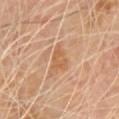| field | value |
|---|---|
| follow-up | no biopsy performed (imaged during a skin exam) |
| lesion size | about 3 mm |
| TBP lesion metrics | a nevus-likeness score of about 0/100 and a lesion-detection confidence of about 100/100 |
| lighting | cross-polarized |
| image source | ~15 mm tile from a whole-body skin photo |
| location | the chest |
| patient | male, aged around 70 |